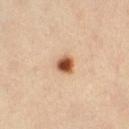{"automated_metrics": {"border_irregularity_0_10": 2.5, "color_variation_0_10": 6.0, "peripheral_color_asymmetry": 1.5}, "image": {"source": "total-body photography crop", "field_of_view_mm": 15}, "site": "right thigh", "lesion_size": {"long_diameter_mm_approx": 2.5}, "patient": {"sex": "male", "age_approx": 40}, "lighting": "cross-polarized"}